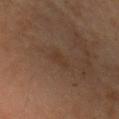Findings:
• biopsy status — total-body-photography surveillance lesion; no biopsy
• image — 15 mm crop, total-body photography
• anatomic site — the arm
• automated metrics — internal color variation of about 0 on a 0–10 scale and peripheral color asymmetry of about 0
• tile lighting — cross-polarized
• diameter — ≈2.5 mm
• subject — female, roughly 70 years of age